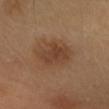| field | value |
|---|---|
| follow-up | imaged on a skin check; not biopsied |
| image source | total-body-photography crop, ~15 mm field of view |
| location | the head or neck |
| lesion diameter | ~5 mm (longest diameter) |
| illumination | cross-polarized illumination |
| subject | female, approximately 40 years of age |